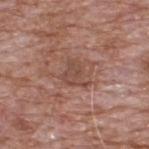Q: Was a biopsy performed?
A: total-body-photography surveillance lesion; no biopsy
Q: What lighting was used for the tile?
A: white-light illumination
Q: What are the patient's age and sex?
A: male, roughly 60 years of age
Q: Lesion size?
A: ≈3.5 mm
Q: What did automated image analysis measure?
A: border irregularity of about 5 on a 0–10 scale, a within-lesion color-variation index near 2/10, and peripheral color asymmetry of about 0.5; a nevus-likeness score of about 0/100 and a detector confidence of about 100 out of 100 that the crop contains a lesion
Q: How was this image acquired?
A: ~15 mm crop, total-body skin-cancer survey
Q: Lesion location?
A: the upper back